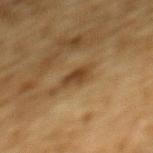Cropped from a total-body skin-imaging series; the visible field is about 15 mm. From the mid back. A male subject about 85 years old or older.